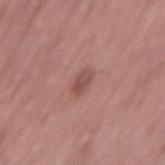Q: Was a biopsy performed?
A: catalogued during a skin exam; not biopsied
Q: Illumination type?
A: white-light
Q: How was this image acquired?
A: ~15 mm tile from a whole-body skin photo
Q: Lesion location?
A: the lower back
Q: What did automated image analysis measure?
A: a mean CIELAB color near L≈50 a*≈22 b*≈24, about 9 CIELAB-L* units darker than the surrounding skin, and a lesion-to-skin contrast of about 6.5 (normalized; higher = more distinct)
Q: How large is the lesion?
A: ≈2.5 mm
Q: What are the patient's age and sex?
A: male, aged 48–52